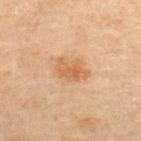Q: What is the anatomic site?
A: the upper back
Q: What are the patient's age and sex?
A: male, in their mid- to late 40s
Q: What did automated image analysis measure?
A: a footprint of about 8 mm² and an eccentricity of roughly 0.75; a lesion color around L≈53 a*≈19 b*≈34 in CIELAB, a lesion–skin lightness drop of about 8, and a lesion-to-skin contrast of about 6.5 (normalized; higher = more distinct)
Q: How was this image acquired?
A: total-body-photography crop, ~15 mm field of view